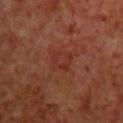Recorded during total-body skin imaging; not selected for excision or biopsy.
The recorded lesion diameter is about 3 mm.
Located on the chest.
A male patient, aged 68–72.
Captured under cross-polarized illumination.
A 15 mm close-up tile from a total-body photography series done for melanoma screening.